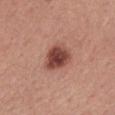| key | value |
|---|---|
| workup | catalogued during a skin exam; not biopsied |
| image | 15 mm crop, total-body photography |
| body site | the back |
| subject | male, roughly 40 years of age |
| automated lesion analysis | an outline eccentricity of about 0.5 (0 = round, 1 = elongated) and a shape-asymmetry score of about 0.2 (0 = symmetric); an average lesion color of about L≈46 a*≈25 b*≈26 (CIELAB), a lesion–skin lightness drop of about 15, and a lesion-to-skin contrast of about 10.5 (normalized; higher = more distinct); a border-irregularity rating of about 2/10, internal color variation of about 5 on a 0–10 scale, and peripheral color asymmetry of about 1.5; a nevus-likeness score of about 90/100 and lesion-presence confidence of about 100/100 |